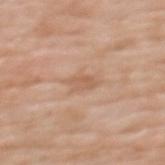No biopsy was performed on this lesion — it was imaged during a full skin examination and was not determined to be concerning. The recorded lesion diameter is about 3.5 mm. An algorithmic analysis of the crop reported a border-irregularity index near 3.5/10, a within-lesion color-variation index near 2/10, and a peripheral color-asymmetry measure near 0.5. The patient is a female aged approximately 70. The tile uses white-light illumination. This image is a 15 mm lesion crop taken from a total-body photograph. From the upper back.